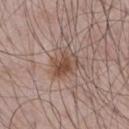{"biopsy_status": "not biopsied; imaged during a skin examination", "patient": {"sex": "male", "age_approx": 65}, "site": "chest", "lighting": "white-light", "image": {"source": "total-body photography crop", "field_of_view_mm": 15}}